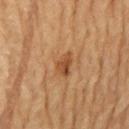Findings:
– biopsy status: imaged on a skin check; not biopsied
– body site: the lower back
– patient: male, aged 83 or older
– acquisition: total-body-photography crop, ~15 mm field of view